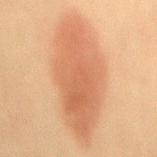| key | value |
|---|---|
| biopsy status | imaged on a skin check; not biopsied |
| body site | the mid back |
| image | ~15 mm tile from a whole-body skin photo |
| subject | male, aged 58 to 62 |
| diameter | about 12.5 mm |